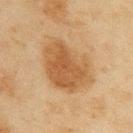Assessment: The lesion was photographed on a routine skin check and not biopsied; there is no pathology result.